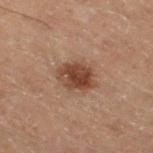imaging modality: ~15 mm crop, total-body skin-cancer survey
image-analysis metrics: an average lesion color of about L≈32 a*≈16 b*≈23 (CIELAB), a lesion–skin lightness drop of about 10, and a lesion-to-skin contrast of about 9.5 (normalized; higher = more distinct); border irregularity of about 1.5 on a 0–10 scale and radial color variation of about 1.5; an automated nevus-likeness rating near 95 out of 100 and a lesion-detection confidence of about 100/100
body site: the right thigh
patient: male, approximately 70 years of age